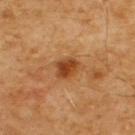  biopsy_status: not biopsied; imaged during a skin examination
  patient:
    sex: male
    age_approx: 60
  automated_metrics:
    vs_skin_darker_L: 11.0
    vs_skin_contrast_norm: 9.0
  image:
    source: total-body photography crop
    field_of_view_mm: 15
  lesion_size:
    long_diameter_mm_approx: 3.0
  lighting: cross-polarized
  site: back